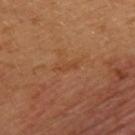<record>
  <biopsy_status>not biopsied; imaged during a skin examination</biopsy_status>
  <image>
    <source>total-body photography crop</source>
    <field_of_view_mm>15</field_of_view_mm>
  </image>
  <site>upper back</site>
  <lesion_size>
    <long_diameter_mm_approx>3.0</long_diameter_mm_approx>
  </lesion_size>
  <lighting>cross-polarized</lighting>
  <patient>
    <sex>male</sex>
    <age_approx>30</age_approx>
  </patient>
</record>